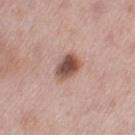Clinical summary: Located on the left thigh. The subject is a female about 50 years old. A 15 mm close-up extracted from a 3D total-body photography capture.